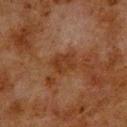Recorded during total-body skin imaging; not selected for excision or biopsy. Imaged with cross-polarized lighting. The patient is a male approximately 80 years of age. Longest diameter approximately 3 mm. A lesion tile, about 15 mm wide, cut from a 3D total-body photograph. Located on the upper back.